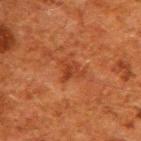Clinical impression:
No biopsy was performed on this lesion — it was imaged during a full skin examination and was not determined to be concerning.
Clinical summary:
On the upper back. A male patient approximately 60 years of age. The total-body-photography lesion software estimated an area of roughly 4.5 mm², an eccentricity of roughly 0.55, and two-axis asymmetry of about 0.5. The software also gave a lesion color around L≈32 a*≈25 b*≈31 in CIELAB, roughly 6 lightness units darker than nearby skin, and a normalized border contrast of about 6. It also reported an automated nevus-likeness rating near 0 out of 100 and a lesion-detection confidence of about 100/100. Approximately 2.5 mm at its widest. A roughly 15 mm field-of-view crop from a total-body skin photograph.